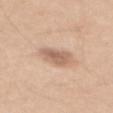A male subject approximately 40 years of age. From the mid back. Captured under white-light illumination. Cropped from a total-body skin-imaging series; the visible field is about 15 mm. The lesion-visualizer software estimated an area of roughly 8 mm² and a shape eccentricity near 0.75. Longest diameter approximately 4 mm.